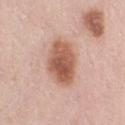Assessment: The lesion was tiled from a total-body skin photograph and was not biopsied. Background: Measured at roughly 5 mm in maximum diameter. Automated tile analysis of the lesion measured a lesion area of about 16 mm², an outline eccentricity of about 0.65 (0 = round, 1 = elongated), and a symmetry-axis asymmetry near 0.15. A female subject aged approximately 65. A 15 mm close-up tile from a total-body photography series done for melanoma screening. On the right thigh. This is a white-light tile.